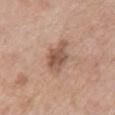Findings:
- biopsy status · imaged on a skin check; not biopsied
- acquisition · total-body-photography crop, ~15 mm field of view
- lighting · white-light
- body site · the chest
- patient · female, aged 58–62